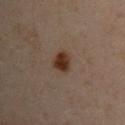  biopsy_status: not biopsied; imaged during a skin examination
  image:
    source: total-body photography crop
    field_of_view_mm: 15
  automated_metrics:
    area_mm2_approx: 4.5
    eccentricity: 0.45
    shape_asymmetry: 0.25
    border_irregularity_0_10: 2.0
    color_variation_0_10: 2.5
    peripheral_color_asymmetry: 1.0
    nevus_likeness_0_100: 100
    lesion_detection_confidence_0_100: 100
  lesion_size:
    long_diameter_mm_approx: 2.5
  lighting: cross-polarized
  site: left arm
  patient:
    sex: male
    age_approx: 50